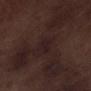Impression:
The lesion was tiled from a total-body skin photograph and was not biopsied.
Context:
The tile uses white-light illumination. Cropped from a total-body skin-imaging series; the visible field is about 15 mm. The subject is a male approximately 70 years of age. The lesion's longest dimension is about 2.5 mm. Located on the left lower leg.